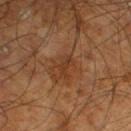Q: Is there a histopathology result?
A: total-body-photography surveillance lesion; no biopsy
Q: What are the patient's age and sex?
A: male, aged approximately 65
Q: Where on the body is the lesion?
A: the left lower leg
Q: How was the tile lit?
A: cross-polarized illumination
Q: What is the imaging modality?
A: ~15 mm tile from a whole-body skin photo